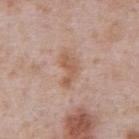Captured during whole-body skin photography for melanoma surveillance; the lesion was not biopsied. Located on the abdomen. A close-up tile cropped from a whole-body skin photograph, about 15 mm across. An algorithmic analysis of the crop reported a lesion area of about 6 mm² and a shape eccentricity near 0.85. The software also gave a detector confidence of about 100 out of 100 that the crop contains a lesion. A male subject aged 63 to 67. The tile uses white-light illumination.